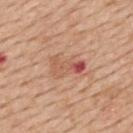The lesion was tiled from a total-body skin photograph and was not biopsied.
The subject is a female in their 50s.
Cropped from a total-body skin-imaging series; the visible field is about 15 mm.
The lesion is on the upper back.
An algorithmic analysis of the crop reported a lesion area of about 8 mm² and an eccentricity of roughly 0.85. The analysis additionally found a mean CIELAB color near L≈57 a*≈25 b*≈31. The software also gave internal color variation of about 9.5 on a 0–10 scale and radial color variation of about 3.
Longest diameter approximately 4 mm.